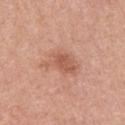<tbp_lesion>
<biopsy_status>not biopsied; imaged during a skin examination</biopsy_status>
<patient>
  <sex>male</sex>
  <age_approx>55</age_approx>
</patient>
<image>
  <source>total-body photography crop</source>
  <field_of_view_mm>15</field_of_view_mm>
</image>
<lesion_size>
  <long_diameter_mm_approx>5.0</long_diameter_mm_approx>
</lesion_size>
<site>chest</site>
</tbp_lesion>